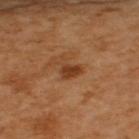<case>
  <biopsy_status>not biopsied; imaged during a skin examination</biopsy_status>
  <image>
    <source>total-body photography crop</source>
    <field_of_view_mm>15</field_of_view_mm>
  </image>
  <site>arm</site>
  <lesion_size>
    <long_diameter_mm_approx>2.5</long_diameter_mm_approx>
  </lesion_size>
  <patient>
    <sex>female</sex>
    <age_approx>55</age_approx>
  </patient>
  <lighting>cross-polarized</lighting>
  <automated_metrics>
    <color_variation_0_10>2.0</color_variation_0_10>
    <peripheral_color_asymmetry>0.5</peripheral_color_asymmetry>
    <nevus_likeness_0_100>40</nevus_likeness_0_100>
    <lesion_detection_confidence_0_100>100</lesion_detection_confidence_0_100>
  </automated_metrics>
</case>